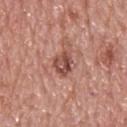Assessment: Part of a total-body skin-imaging series; this lesion was reviewed on a skin check and was not flagged for biopsy. Context: The patient is a male in their mid- to late 70s. Captured under white-light illumination. Longest diameter approximately 3.5 mm. The total-body-photography lesion software estimated an area of roughly 5 mm², an eccentricity of roughly 0.75, and two-axis asymmetry of about 0.25. The software also gave a lesion color around L≈49 a*≈24 b*≈26 in CIELAB, about 12 CIELAB-L* units darker than the surrounding skin, and a normalized lesion–skin contrast near 8.5. It also reported a border-irregularity index near 2.5/10, internal color variation of about 6 on a 0–10 scale, and radial color variation of about 2. Located on the upper back. This image is a 15 mm lesion crop taken from a total-body photograph.